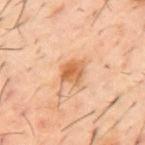Clinical impression: The lesion was tiled from a total-body skin photograph and was not biopsied. Acquisition and patient details: On the mid back. The lesion-visualizer software estimated an average lesion color of about L≈58 a*≈23 b*≈38 (CIELAB), roughly 11 lightness units darker than nearby skin, and a normalized lesion–skin contrast near 8. The software also gave an automated nevus-likeness rating near 80 out of 100 and a lesion-detection confidence of about 100/100. A 15 mm close-up tile from a total-body photography series done for melanoma screening. A male subject in their 60s. This is a cross-polarized tile.